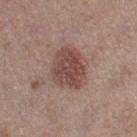Part of a total-body skin-imaging series; this lesion was reviewed on a skin check and was not flagged for biopsy.
The lesion's longest dimension is about 5 mm.
The lesion is located on the left thigh.
The subject is a female about 40 years old.
Cropped from a whole-body photographic skin survey; the tile spans about 15 mm.